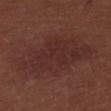{
  "biopsy_status": "not biopsied; imaged during a skin examination",
  "lesion_size": {
    "long_diameter_mm_approx": 10.5
  },
  "automated_metrics": {
    "area_mm2_approx": 40.0,
    "eccentricity": 0.85,
    "shape_asymmetry": 0.25
  },
  "lighting": "white-light",
  "site": "upper back",
  "image": {
    "source": "total-body photography crop",
    "field_of_view_mm": 15
  },
  "patient": {
    "sex": "male",
    "age_approx": 30
  }
}